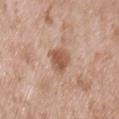This lesion was catalogued during total-body skin photography and was not selected for biopsy.
Captured under white-light illumination.
On the chest.
The total-body-photography lesion software estimated a nevus-likeness score of about 55/100 and lesion-presence confidence of about 100/100.
A close-up tile cropped from a whole-body skin photograph, about 15 mm across.
A male subject, aged 48–52.